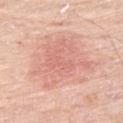Case summary:
– follow-up: no biopsy performed (imaged during a skin exam)
– illumination: white-light
– imaging modality: 15 mm crop, total-body photography
– patient: male, approximately 80 years of age
– site: the right thigh
– image-analysis metrics: an area of roughly 27 mm², an eccentricity of roughly 0.55, and two-axis asymmetry of about 0.35; a within-lesion color-variation index near 3.5/10